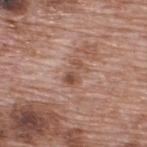notes: catalogued during a skin exam; not biopsied
patient: male, approximately 70 years of age
tile lighting: white-light
image source: ~15 mm tile from a whole-body skin photo
automated metrics: a lesion color around L≈51 a*≈21 b*≈28 in CIELAB, roughly 9 lightness units darker than nearby skin, and a normalized border contrast of about 7; a border-irregularity index near 5/10
body site: the back